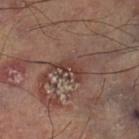This lesion was catalogued during total-body skin photography and was not selected for biopsy. The lesion is located on the leg. About 4.5 mm across. The tile uses cross-polarized illumination. This image is a 15 mm lesion crop taken from a total-body photograph. An algorithmic analysis of the crop reported a lesion color around L≈41 a*≈19 b*≈23 in CIELAB, a lesion–skin lightness drop of about 7, and a normalized lesion–skin contrast near 6. The software also gave internal color variation of about 4 on a 0–10 scale and peripheral color asymmetry of about 1.5.